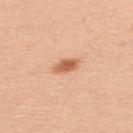Q: Was this lesion biopsied?
A: imaged on a skin check; not biopsied
Q: Automated lesion metrics?
A: an average lesion color of about L≈62 a*≈25 b*≈37 (CIELAB), a lesion–skin lightness drop of about 13, and a lesion-to-skin contrast of about 8.5 (normalized; higher = more distinct); a classifier nevus-likeness of about 100/100
Q: How was this image acquired?
A: 15 mm crop, total-body photography
Q: Patient demographics?
A: male, in their mid-40s
Q: How was the tile lit?
A: white-light
Q: Lesion size?
A: ≈3 mm
Q: Lesion location?
A: the back